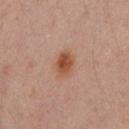Part of a total-body skin-imaging series; this lesion was reviewed on a skin check and was not flagged for biopsy. The lesion is located on the left upper arm. The lesion-visualizer software estimated an average lesion color of about L≈49 a*≈22 b*≈31 (CIELAB), about 9 CIELAB-L* units darker than the surrounding skin, and a normalized border contrast of about 8.5. It also reported border irregularity of about 2 on a 0–10 scale, a within-lesion color-variation index near 5/10, and peripheral color asymmetry of about 1.5. Cropped from a total-body skin-imaging series; the visible field is about 15 mm. Imaged with cross-polarized lighting. The recorded lesion diameter is about 3.5 mm. A male subject in their mid- to late 30s.